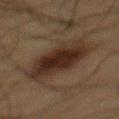Imaged during a routine full-body skin examination; the lesion was not biopsied and no histopathology is available.
The tile uses cross-polarized illumination.
This image is a 15 mm lesion crop taken from a total-body photograph.
A male patient, roughly 60 years of age.
Measured at roughly 8 mm in maximum diameter.
From the mid back.
An algorithmic analysis of the crop reported border irregularity of about 3 on a 0–10 scale, internal color variation of about 4.5 on a 0–10 scale, and a peripheral color-asymmetry measure near 1.